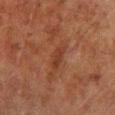A 15 mm crop from a total-body photograph taken for skin-cancer surveillance. The lesion is on the left lower leg. A male subject aged approximately 80.